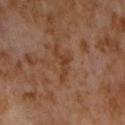The tile uses cross-polarized illumination.
A 15 mm close-up tile from a total-body photography series done for melanoma screening.
The recorded lesion diameter is about 4.5 mm.
A male subject, approximately 60 years of age.
The lesion is located on the chest.